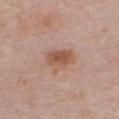Case summary:
- biopsy status: catalogued during a skin exam; not biopsied
- image: total-body-photography crop, ~15 mm field of view
- patient: female, aged approximately 50
- lesion diameter: about 3.5 mm
- location: the front of the torso
- lighting: white-light